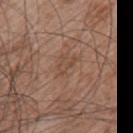biopsy status: catalogued during a skin exam; not biopsied | body site: the upper back | acquisition: ~15 mm crop, total-body skin-cancer survey | patient: male, in their mid-60s | tile lighting: white-light illumination.